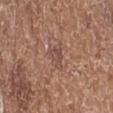automated lesion analysis: a mean CIELAB color near L≈48 a*≈20 b*≈25 and a lesion-to-skin contrast of about 5.5 (normalized; higher = more distinct); an automated nevus-likeness rating near 0 out of 100 and a lesion-detection confidence of about 95/100 | anatomic site: the leg | illumination: white-light illumination | size: ~3 mm (longest diameter) | image: ~15 mm tile from a whole-body skin photo | patient: female, approximately 75 years of age.